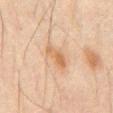biopsy status = catalogued during a skin exam; not biopsied | lesion size = ≈3.5 mm | body site = the mid back | patient = male, roughly 70 years of age | automated lesion analysis = a lesion color around L≈53 a*≈17 b*≈32 in CIELAB | imaging modality = total-body-photography crop, ~15 mm field of view | illumination = cross-polarized.